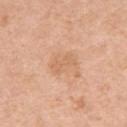Impression: Imaged during a routine full-body skin examination; the lesion was not biopsied and no histopathology is available. Background: On the left upper arm. The lesion's longest dimension is about 3 mm. Automated tile analysis of the lesion measured a mean CIELAB color near L≈65 a*≈22 b*≈36, about 6 CIELAB-L* units darker than the surrounding skin, and a normalized border contrast of about 4.5. The software also gave a classifier nevus-likeness of about 0/100 and a detector confidence of about 100 out of 100 that the crop contains a lesion. A 15 mm close-up extracted from a 3D total-body photography capture. The subject is a female in their 40s.